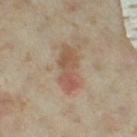| feature | finding |
|---|---|
| notes | no biopsy performed (imaged during a skin exam) |